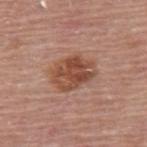Imaged during a routine full-body skin examination; the lesion was not biopsied and no histopathology is available. A male subject roughly 80 years of age. An algorithmic analysis of the crop reported a footprint of about 14 mm², an eccentricity of roughly 0.7, and a symmetry-axis asymmetry near 0.15. The analysis additionally found a border-irregularity rating of about 2/10, a within-lesion color-variation index near 5.5/10, and a peripheral color-asymmetry measure near 2. The analysis additionally found an automated nevus-likeness rating near 60 out of 100. About 5 mm across. A 15 mm close-up extracted from a 3D total-body photography capture. Captured under white-light illumination. On the mid back.